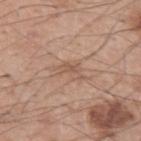biopsy status: imaged on a skin check; not biopsied | subject: male, roughly 55 years of age | acquisition: total-body-photography crop, ~15 mm field of view.